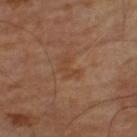Assessment:
Part of a total-body skin-imaging series; this lesion was reviewed on a skin check and was not flagged for biopsy.
Background:
A male subject in their mid-60s. An algorithmic analysis of the crop reported border irregularity of about 6 on a 0–10 scale and a peripheral color-asymmetry measure near 0. The software also gave an automated nevus-likeness rating near 0 out of 100 and lesion-presence confidence of about 100/100. A roughly 15 mm field-of-view crop from a total-body skin photograph. The tile uses cross-polarized illumination. The lesion's longest dimension is about 3 mm.